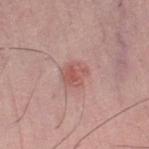follow-up — catalogued during a skin exam; not biopsied | illumination — white-light illumination | image — 15 mm crop, total-body photography | location — the right thigh | patient — male, approximately 50 years of age.